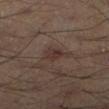| feature | finding |
|---|---|
| biopsy status | catalogued during a skin exam; not biopsied |
| body site | the right lower leg |
| patient | male, in their mid-50s |
| acquisition | 15 mm crop, total-body photography |
| illumination | cross-polarized |
| automated metrics | an average lesion color of about L≈30 a*≈15 b*≈18 (CIELAB), about 6 CIELAB-L* units darker than the surrounding skin, and a normalized lesion–skin contrast near 7; border irregularity of about 3.5 on a 0–10 scale, a color-variation rating of about 2.5/10, and peripheral color asymmetry of about 1; an automated nevus-likeness rating near 15 out of 100 |